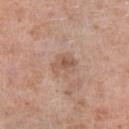workup = total-body-photography surveillance lesion; no biopsy
TBP lesion metrics = an area of roughly 5.5 mm² and a symmetry-axis asymmetry near 0.35; an average lesion color of about L≈56 a*≈19 b*≈29 (CIELAB) and a normalized border contrast of about 6; border irregularity of about 3.5 on a 0–10 scale, a color-variation rating of about 4/10, and peripheral color asymmetry of about 1.5; a detector confidence of about 100 out of 100 that the crop contains a lesion
lighting = white-light
imaging modality = total-body-photography crop, ~15 mm field of view
diameter = ~3 mm (longest diameter)
subject = male, in their 80s
location = the left lower leg